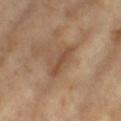biopsy status = no biopsy performed (imaged during a skin exam); location = the right thigh; image = 15 mm crop, total-body photography; patient = female, aged 73 to 77.